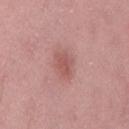biopsy status = imaged on a skin check; not biopsied
site = the leg
illumination = white-light illumination
subject = female, aged around 50
image source = 15 mm crop, total-body photography
lesion size = ~3.5 mm (longest diameter)